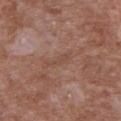patient: male, in their mid- to late 40s | tile lighting: white-light illumination | location: the front of the torso | TBP lesion metrics: a lesion area of about 3 mm², a shape eccentricity near 0.95, and a shape-asymmetry score of about 0.35 (0 = symmetric); an automated nevus-likeness rating near 0 out of 100 | diameter: about 3.5 mm | acquisition: total-body-photography crop, ~15 mm field of view.